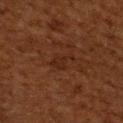This lesion was catalogued during total-body skin photography and was not selected for biopsy. A male patient roughly 60 years of age. On the arm. A region of skin cropped from a whole-body photographic capture, roughly 15 mm wide.